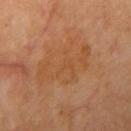| field | value |
|---|---|
| biopsy status | catalogued during a skin exam; not biopsied |
| lighting | cross-polarized illumination |
| TBP lesion metrics | a lesion color around L≈48 a*≈22 b*≈36 in CIELAB, about 5 CIELAB-L* units darker than the surrounding skin, and a normalized border contrast of about 5; radial color variation of about 1; a nevus-likeness score of about 0/100 and lesion-presence confidence of about 100/100 |
| subject | female, about 70 years old |
| imaging modality | total-body-photography crop, ~15 mm field of view |
| size | ≈7.5 mm |
| site | the right upper arm |